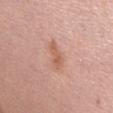Assessment: No biopsy was performed on this lesion — it was imaged during a full skin examination and was not determined to be concerning.